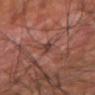Background: From the arm. This is a cross-polarized tile. Automated tile analysis of the lesion measured a border-irregularity index near 3.5/10 and radial color variation of about 0. About 2.5 mm across. A region of skin cropped from a whole-body photographic capture, roughly 15 mm wide. A male patient, aged around 60.